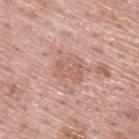Q: Was a biopsy performed?
A: catalogued during a skin exam; not biopsied
Q: What is the imaging modality?
A: total-body-photography crop, ~15 mm field of view
Q: Lesion location?
A: the back
Q: Who is the patient?
A: male, aged 73–77